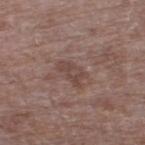This lesion was catalogued during total-body skin photography and was not selected for biopsy.